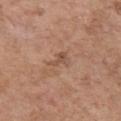Q: Was this lesion biopsied?
A: total-body-photography surveillance lesion; no biopsy
Q: What is the lesion's diameter?
A: ≈2.5 mm
Q: Illumination type?
A: white-light illumination
Q: What are the patient's age and sex?
A: female, aged 63–67
Q: How was this image acquired?
A: ~15 mm tile from a whole-body skin photo
Q: Where on the body is the lesion?
A: the left upper arm